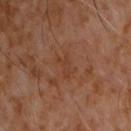Notes:
– follow-up · catalogued during a skin exam; not biopsied
– lighting · cross-polarized illumination
– automated metrics · a border-irregularity rating of about 5.5/10, internal color variation of about 0 on a 0–10 scale, and radial color variation of about 0
– lesion diameter · about 3 mm
– subject · male, aged 58–62
– imaging modality · total-body-photography crop, ~15 mm field of view
– anatomic site · the chest